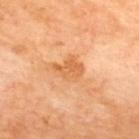Q: How large is the lesion?
A: about 3.5 mm
Q: How was the tile lit?
A: cross-polarized illumination
Q: Where on the body is the lesion?
A: the back
Q: What did automated image analysis measure?
A: internal color variation of about 3 on a 0–10 scale and a peripheral color-asymmetry measure near 1
Q: What are the patient's age and sex?
A: male, aged around 70
Q: How was this image acquired?
A: ~15 mm tile from a whole-body skin photo